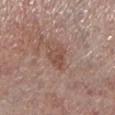Notes:
- notes — catalogued during a skin exam; not biopsied
- site — the leg
- image — 15 mm crop, total-body photography
- tile lighting — white-light
- subject — female, approximately 65 years of age
- diameter — ~3 mm (longest diameter)